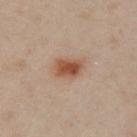Q: Is there a histopathology result?
A: total-body-photography surveillance lesion; no biopsy
Q: How was the tile lit?
A: cross-polarized
Q: How was this image acquired?
A: 15 mm crop, total-body photography
Q: Automated lesion metrics?
A: a lesion area of about 6.5 mm², a shape eccentricity near 0.6, and two-axis asymmetry of about 0.25; a border-irregularity rating of about 2/10 and peripheral color asymmetry of about 1.5
Q: Lesion location?
A: the arm
Q: Who is the patient?
A: female, aged approximately 40